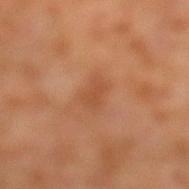Case summary:
• workup · catalogued during a skin exam; not biopsied
• lighting · cross-polarized illumination
• automated metrics · an area of roughly 4 mm², an outline eccentricity of about 0.85 (0 = round, 1 = elongated), and a shape-asymmetry score of about 0.3 (0 = symmetric)
• diameter · ~3 mm (longest diameter)
• body site · the left lower leg
• image source · total-body-photography crop, ~15 mm field of view
• patient · male, roughly 30 years of age